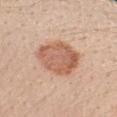Q: Was this lesion biopsied?
A: catalogued during a skin exam; not biopsied